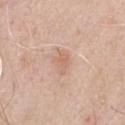From the chest.
Approximately 3 mm at its widest.
A 15 mm close-up extracted from a 3D total-body photography capture.
This is a white-light tile.
The patient is a male in their 70s.
Automated tile analysis of the lesion measured a lesion color around L≈64 a*≈20 b*≈29 in CIELAB, a lesion–skin lightness drop of about 7, and a normalized lesion–skin contrast near 5. It also reported an automated nevus-likeness rating near 0 out of 100 and lesion-presence confidence of about 100/100.